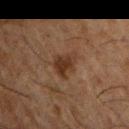workup: catalogued during a skin exam; not biopsied
subject: male, roughly 50 years of age
lesion size: about 3 mm
anatomic site: the front of the torso
image: total-body-photography crop, ~15 mm field of view